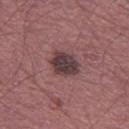Captured during whole-body skin photography for melanoma surveillance; the lesion was not biopsied.
The tile uses white-light illumination.
Longest diameter approximately 3.5 mm.
A roughly 15 mm field-of-view crop from a total-body skin photograph.
The subject is a male aged around 60.
On the left thigh.